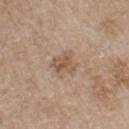Captured under white-light illumination.
The lesion is located on the chest.
The subject is a male approximately 65 years of age.
Cropped from a whole-body photographic skin survey; the tile spans about 15 mm.
Automated image analysis of the tile measured border irregularity of about 3 on a 0–10 scale, a within-lesion color-variation index near 4/10, and peripheral color asymmetry of about 1.5.
Longest diameter approximately 3.5 mm.